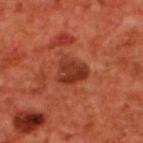| key | value |
|---|---|
| workup | imaged on a skin check; not biopsied |
| patient | male, aged 68 to 72 |
| tile lighting | cross-polarized illumination |
| image source | 15 mm crop, total-body photography |
| diameter | ≈3.5 mm |
| location | the back |